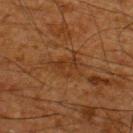lesion diameter = about 5 mm; patient = male, in their mid- to late 60s; location = the back; image source = total-body-photography crop, ~15 mm field of view; lighting = cross-polarized.